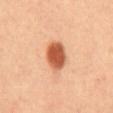Q: Was this lesion biopsied?
A: imaged on a skin check; not biopsied
Q: Patient demographics?
A: male, in their 40s
Q: What is the anatomic site?
A: the back
Q: What is the lesion's diameter?
A: ≈4.5 mm
Q: Automated lesion metrics?
A: roughly 18 lightness units darker than nearby skin and a normalized border contrast of about 11; a peripheral color-asymmetry measure near 1
Q: What kind of image is this?
A: 15 mm crop, total-body photography